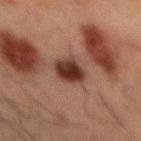Case summary:
* image — 15 mm crop, total-body photography
* subject — male, approximately 50 years of age
* illumination — cross-polarized
* anatomic site — the mid back
* lesion diameter — about 4 mm